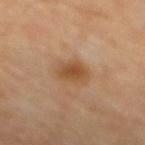follow-up=catalogued during a skin exam; not biopsied
lesion size=~3 mm (longest diameter)
automated metrics=a lesion color around L≈48 a*≈20 b*≈35 in CIELAB and a lesion–skin lightness drop of about 9
subject=aged around 65
location=the mid back
image source=15 mm crop, total-body photography